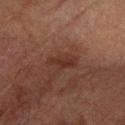Q: Is there a histopathology result?
A: imaged on a skin check; not biopsied
Q: What did automated image analysis measure?
A: a shape eccentricity near 0.85 and a symmetry-axis asymmetry near 0.35; roughly 6 lightness units darker than nearby skin; a border-irregularity index near 4/10, a color-variation rating of about 2/10, and peripheral color asymmetry of about 1; a nevus-likeness score of about 15/100 and a detector confidence of about 100 out of 100 that the crop contains a lesion
Q: What is the anatomic site?
A: the right forearm
Q: What is the imaging modality?
A: 15 mm crop, total-body photography
Q: How large is the lesion?
A: ~3.5 mm (longest diameter)
Q: Who is the patient?
A: male, aged around 75
Q: What lighting was used for the tile?
A: cross-polarized illumination